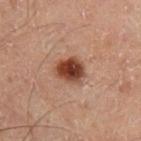Notes:
* notes · imaged on a skin check; not biopsied
* location · the right lower leg
* subject · male, roughly 70 years of age
* diameter · ≈3.5 mm
* illumination · cross-polarized
* acquisition · ~15 mm crop, total-body skin-cancer survey